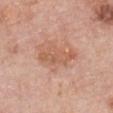Impression: Captured during whole-body skin photography for melanoma surveillance; the lesion was not biopsied. Image and clinical context: Located on the chest. The tile uses white-light illumination. A region of skin cropped from a whole-body photographic capture, roughly 15 mm wide. A female subject, in their 60s. Measured at roughly 5 mm in maximum diameter.